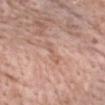Clinical impression: Imaged during a routine full-body skin examination; the lesion was not biopsied and no histopathology is available. Image and clinical context: Cropped from a whole-body photographic skin survey; the tile spans about 15 mm. From the front of the torso. Approximately 3.5 mm at its widest. The patient is a female approximately 75 years of age. Imaged with white-light lighting.